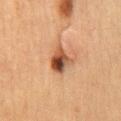The lesion was photographed on a routine skin check and not biopsied; there is no pathology result.